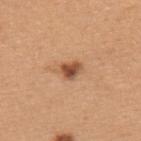Notes:
- notes · total-body-photography surveillance lesion; no biopsy
- size · ~3 mm (longest diameter)
- subject · female, approximately 45 years of age
- automated metrics · border irregularity of about 3 on a 0–10 scale and peripheral color asymmetry of about 1.5; a classifier nevus-likeness of about 90/100 and lesion-presence confidence of about 100/100
- site · the upper back
- illumination · white-light
- imaging modality · ~15 mm tile from a whole-body skin photo